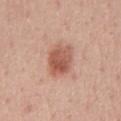Q: Was this lesion biopsied?
A: imaged on a skin check; not biopsied
Q: How was this image acquired?
A: total-body-photography crop, ~15 mm field of view
Q: Who is the patient?
A: male, approximately 50 years of age
Q: Lesion location?
A: the chest